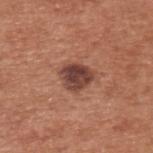| field | value |
|---|---|
| biopsy status | imaged on a skin check; not biopsied |
| anatomic site | the back |
| acquisition | 15 mm crop, total-body photography |
| patient | male, aged approximately 55 |
| lighting | white-light illumination |
| size | ≈3.5 mm |
| TBP lesion metrics | a footprint of about 8.5 mm², an eccentricity of roughly 0.5, and a symmetry-axis asymmetry near 0.25; an average lesion color of about L≈42 a*≈23 b*≈25 (CIELAB), about 14 CIELAB-L* units darker than the surrounding skin, and a normalized lesion–skin contrast near 11 |